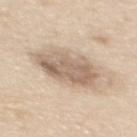biopsy status = no biopsy performed (imaged during a skin exam) | image source = total-body-photography crop, ~15 mm field of view | tile lighting = white-light | lesion size = ~6 mm (longest diameter) | site = the mid back | patient = female, aged around 55.